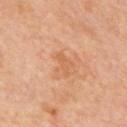Q: Was this lesion biopsied?
A: imaged on a skin check; not biopsied
Q: Patient demographics?
A: male, aged 58–62
Q: How was this image acquired?
A: ~15 mm crop, total-body skin-cancer survey
Q: Where on the body is the lesion?
A: the upper back